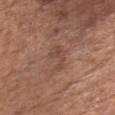This lesion was catalogued during total-body skin photography and was not selected for biopsy.
The lesion is located on the chest.
A male patient, approximately 65 years of age.
A roughly 15 mm field-of-view crop from a total-body skin photograph.